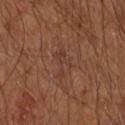Captured during whole-body skin photography for melanoma surveillance; the lesion was not biopsied.